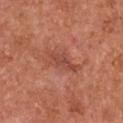biopsy status: no biopsy performed (imaged during a skin exam); tile lighting: white-light; automated lesion analysis: a lesion area of about 7 mm², an eccentricity of roughly 0.9, and two-axis asymmetry of about 0.4; subject: female, aged 48–52; imaging modality: total-body-photography crop, ~15 mm field of view; location: the chest.